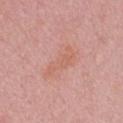<record>
  <biopsy_status>not biopsied; imaged during a skin examination</biopsy_status>
  <lighting>white-light</lighting>
  <lesion_size>
    <long_diameter_mm_approx>4.5</long_diameter_mm_approx>
  </lesion_size>
  <image>
    <source>total-body photography crop</source>
    <field_of_view_mm>15</field_of_view_mm>
  </image>
  <site>left upper arm</site>
  <patient>
    <sex>male</sex>
    <age_approx>50</age_approx>
  </patient>
</record>